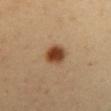follow-up: total-body-photography surveillance lesion; no biopsy
subject: female, roughly 55 years of age
acquisition: total-body-photography crop, ~15 mm field of view
TBP lesion metrics: border irregularity of about 1 on a 0–10 scale, internal color variation of about 4.5 on a 0–10 scale, and radial color variation of about 1.5
illumination: cross-polarized
anatomic site: the chest
lesion size: about 3 mm